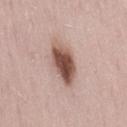Q: Was a biopsy performed?
A: no biopsy performed (imaged during a skin exam)
Q: How large is the lesion?
A: about 5 mm
Q: What is the imaging modality?
A: 15 mm crop, total-body photography
Q: What did automated image analysis measure?
A: a lesion area of about 11 mm², an outline eccentricity of about 0.85 (0 = round, 1 = elongated), and a shape-asymmetry score of about 0.2 (0 = symmetric); an average lesion color of about L≈51 a*≈20 b*≈24 (CIELAB), roughly 18 lightness units darker than nearby skin, and a normalized lesion–skin contrast near 12; border irregularity of about 2.5 on a 0–10 scale, a color-variation rating of about 6.5/10, and a peripheral color-asymmetry measure near 2.5
Q: Patient demographics?
A: female, aged approximately 30
Q: What lighting was used for the tile?
A: white-light
Q: Lesion location?
A: the lower back